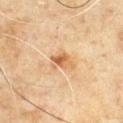biopsy status: imaged on a skin check; not biopsied | image: ~15 mm tile from a whole-body skin photo | automated lesion analysis: a footprint of about 4.5 mm² and a shape-asymmetry score of about 0.3 (0 = symmetric); an automated nevus-likeness rating near 20 out of 100 and lesion-presence confidence of about 100/100 | subject: male, aged 63 to 67 | diameter: ~3 mm (longest diameter) | site: the chest | tile lighting: cross-polarized.